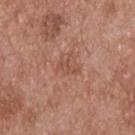Impression: The lesion was photographed on a routine skin check and not biopsied; there is no pathology result. Clinical summary: On the upper back. Imaged with white-light lighting. Automated tile analysis of the lesion measured an area of roughly 3 mm² and a shape eccentricity near 0.85. It also reported a lesion color around L≈51 a*≈23 b*≈29 in CIELAB, a lesion–skin lightness drop of about 6, and a normalized lesion–skin contrast near 5. The lesion's longest dimension is about 3 mm. A roughly 15 mm field-of-view crop from a total-body skin photograph. The patient is a male in their mid- to late 50s.